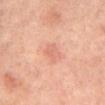notes — no biopsy performed (imaged during a skin exam) | lesion diameter — ~3 mm (longest diameter) | subject — male, in their mid-60s | image source — total-body-photography crop, ~15 mm field of view | body site — the mid back | illumination — cross-polarized illumination | TBP lesion metrics — a shape-asymmetry score of about 0.3 (0 = symmetric); a classifier nevus-likeness of about 0/100 and a lesion-detection confidence of about 100/100.